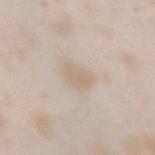{"biopsy_status": "not biopsied; imaged during a skin examination", "site": "left forearm", "lesion_size": {"long_diameter_mm_approx": 3.0}, "automated_metrics": {"area_mm2_approx": 6.5, "eccentricity": 0.6, "shape_asymmetry": 0.3, "vs_skin_darker_L": 6.0, "vs_skin_contrast_norm": 5.0, "nevus_likeness_0_100": 0}, "patient": {"sex": "female", "age_approx": 25}, "image": {"source": "total-body photography crop", "field_of_view_mm": 15}, "lighting": "white-light"}